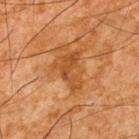Impression: No biopsy was performed on this lesion — it was imaged during a full skin examination and was not determined to be concerning. Clinical summary: A male patient, aged approximately 65. Captured under cross-polarized illumination. Cropped from a total-body skin-imaging series; the visible field is about 15 mm. On the back. Approximately 4.5 mm at its widest.